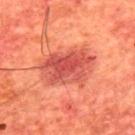Assessment: The lesion was tiled from a total-body skin photograph and was not biopsied. Acquisition and patient details: A roughly 15 mm field-of-view crop from a total-body skin photograph. A male subject in their mid- to late 60s. About 6 mm across. This is a cross-polarized tile. The lesion is on the upper back. Automated tile analysis of the lesion measured a footprint of about 19 mm², a shape eccentricity near 0.75, and two-axis asymmetry of about 0.2. It also reported a lesion color around L≈49 a*≈36 b*≈31 in CIELAB. The software also gave a border-irregularity index near 2.5/10 and peripheral color asymmetry of about 2. The analysis additionally found a classifier nevus-likeness of about 90/100 and a lesion-detection confidence of about 100/100.